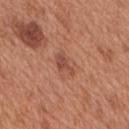Captured under white-light illumination. A 15 mm close-up extracted from a 3D total-body photography capture. Automated tile analysis of the lesion measured a classifier nevus-likeness of about 5/100 and a detector confidence of about 100 out of 100 that the crop contains a lesion. From the back. A male patient roughly 65 years of age. Measured at roughly 3 mm in maximum diameter.